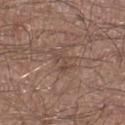Clinical impression:
No biopsy was performed on this lesion — it was imaged during a full skin examination and was not determined to be concerning.
Clinical summary:
A lesion tile, about 15 mm wide, cut from a 3D total-body photograph. Located on the leg. A male patient, roughly 50 years of age.